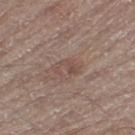Assessment: The lesion was tiled from a total-body skin photograph and was not biopsied. Acquisition and patient details: A region of skin cropped from a whole-body photographic capture, roughly 15 mm wide. A male patient aged approximately 65. From the left thigh.